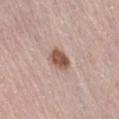Q: Is there a histopathology result?
A: catalogued during a skin exam; not biopsied
Q: How was the tile lit?
A: white-light
Q: How large is the lesion?
A: ≈3 mm
Q: Lesion location?
A: the abdomen
Q: Who is the patient?
A: female, aged 63 to 67
Q: How was this image acquired?
A: total-body-photography crop, ~15 mm field of view